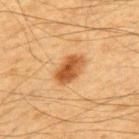Assessment: Recorded during total-body skin imaging; not selected for excision or biopsy. Context: A 15 mm crop from a total-body photograph taken for skin-cancer surveillance. The recorded lesion diameter is about 4 mm. A male patient, aged approximately 65. Located on the back. Automated image analysis of the tile measured an area of roughly 8 mm², an outline eccentricity of about 0.8 (0 = round, 1 = elongated), and two-axis asymmetry of about 0.15. The software also gave a classifier nevus-likeness of about 100/100 and a detector confidence of about 100 out of 100 that the crop contains a lesion. Captured under cross-polarized illumination.